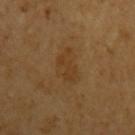biopsy_status: not biopsied; imaged during a skin examination
site: left upper arm
automated_metrics:
  eccentricity: 0.8
  shape_asymmetry: 0.25
  cielab_L: 38
  cielab_a: 17
  cielab_b: 34
  vs_skin_darker_L: 5.0
  border_irregularity_0_10: 3.0
  color_variation_0_10: 2.5
  peripheral_color_asymmetry: 1.0
  nevus_likeness_0_100: 0
  lesion_detection_confidence_0_100: 100
lighting: cross-polarized
patient:
  sex: male
  age_approx: 65
image:
  source: total-body photography crop
  field_of_view_mm: 15